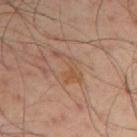Clinical impression:
Recorded during total-body skin imaging; not selected for excision or biopsy.
Image and clinical context:
The total-body-photography lesion software estimated an area of roughly 6.5 mm², an outline eccentricity of about 0.9 (0 = round, 1 = elongated), and two-axis asymmetry of about 0.6. The software also gave a lesion–skin lightness drop of about 6. And it measured border irregularity of about 8 on a 0–10 scale, a color-variation rating of about 2/10, and peripheral color asymmetry of about 1. The patient is a male about 50 years old. The lesion is on the arm. The tile uses cross-polarized illumination. Measured at roughly 5 mm in maximum diameter. A 15 mm close-up extracted from a 3D total-body photography capture.